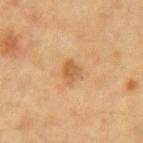biopsy status — no biopsy performed (imaged during a skin exam) | tile lighting — cross-polarized | subject — female, roughly 40 years of age | image source — ~15 mm crop, total-body skin-cancer survey | body site — the left upper arm.